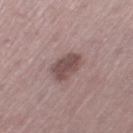Impression:
The lesion was photographed on a routine skin check and not biopsied; there is no pathology result.
Clinical summary:
A 15 mm close-up extracted from a 3D total-body photography capture. On the left thigh. The patient is a female aged around 45.